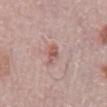Clinical impression:
The lesion was tiled from a total-body skin photograph and was not biopsied.
Context:
The patient is a male in their mid- to late 70s. On the mid back. This is a white-light tile. The total-body-photography lesion software estimated an average lesion color of about L≈56 a*≈22 b*≈24 (CIELAB), a lesion–skin lightness drop of about 9, and a normalized lesion–skin contrast near 6.5. A lesion tile, about 15 mm wide, cut from a 3D total-body photograph. The lesion's longest dimension is about 2.5 mm.